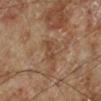Imaged during a routine full-body skin examination; the lesion was not biopsied and no histopathology is available. On the left lower leg. A 15 mm close-up extracted from a 3D total-body photography capture. Longest diameter approximately 2.5 mm. The total-body-photography lesion software estimated an average lesion color of about L≈43 a*≈18 b*≈30 (CIELAB), a lesion–skin lightness drop of about 7, and a lesion-to-skin contrast of about 5.5 (normalized; higher = more distinct). The analysis additionally found a border-irregularity rating of about 4/10, a color-variation rating of about 1/10, and a peripheral color-asymmetry measure near 0. It also reported a nevus-likeness score of about 0/100 and lesion-presence confidence of about 100/100. A male patient aged 68 to 72. Imaged with cross-polarized lighting.